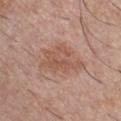This lesion was catalogued during total-body skin photography and was not selected for biopsy. The total-body-photography lesion software estimated a nevus-likeness score of about 10/100 and lesion-presence confidence of about 100/100. The lesion is on the chest. The subject is a male aged approximately 30. A roughly 15 mm field-of-view crop from a total-body skin photograph. The recorded lesion diameter is about 5.5 mm. The tile uses white-light illumination.